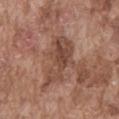This lesion was catalogued during total-body skin photography and was not selected for biopsy.
Measured at roughly 6.5 mm in maximum diameter.
A male patient, aged around 75.
A lesion tile, about 15 mm wide, cut from a 3D total-body photograph.
The lesion is on the front of the torso.